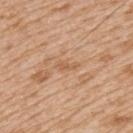Captured under white-light illumination. A lesion tile, about 15 mm wide, cut from a 3D total-body photograph. On the left upper arm. An algorithmic analysis of the crop reported an outline eccentricity of about 0.9 (0 = round, 1 = elongated) and two-axis asymmetry of about 0.4. It also reported a mean CIELAB color near L≈59 a*≈20 b*≈36, about 7 CIELAB-L* units darker than the surrounding skin, and a lesion-to-skin contrast of about 5 (normalized; higher = more distinct). The software also gave border irregularity of about 4 on a 0–10 scale and peripheral color asymmetry of about 0.5. It also reported a nevus-likeness score of about 0/100 and lesion-presence confidence of about 70/100. The subject is a male aged approximately 65. The lesion's longest dimension is about 3 mm.